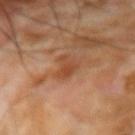Impression: The lesion was tiled from a total-body skin photograph and was not biopsied.